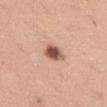The subject is a female aged around 45. A 15 mm close-up tile from a total-body photography series done for melanoma screening. This is a white-light tile. The lesion is located on the left thigh. Longest diameter approximately 3 mm.